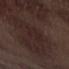{"patient": {"sex": "male", "age_approx": 70}, "image": {"source": "total-body photography crop", "field_of_view_mm": 15}, "lesion_size": {"long_diameter_mm_approx": 4.5}, "site": "arm", "lighting": "white-light"}